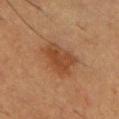Clinical impression:
No biopsy was performed on this lesion — it was imaged during a full skin examination and was not determined to be concerning.
Clinical summary:
Measured at roughly 4.5 mm in maximum diameter. This is a cross-polarized tile. From the upper back. The patient is a male aged 53–57. A roughly 15 mm field-of-view crop from a total-body skin photograph.